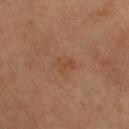A female subject, approximately 60 years of age.
A 15 mm close-up tile from a total-body photography series done for melanoma screening.
Located on the left leg.